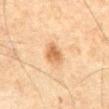Q: Was this lesion biopsied?
A: total-body-photography surveillance lesion; no biopsy
Q: How large is the lesion?
A: ~3 mm (longest diameter)
Q: Lesion location?
A: the abdomen
Q: What kind of image is this?
A: ~15 mm tile from a whole-body skin photo
Q: How was the tile lit?
A: cross-polarized
Q: Patient demographics?
A: male, aged 63–67